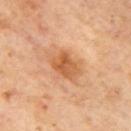<case>
  <biopsy_status>not biopsied; imaged during a skin examination</biopsy_status>
  <image>
    <source>total-body photography crop</source>
    <field_of_view_mm>15</field_of_view_mm>
  </image>
  <lesion_size>
    <long_diameter_mm_approx>4.0</long_diameter_mm_approx>
  </lesion_size>
  <site>right upper arm</site>
  <patient>
    <sex>female</sex>
    <age_approx>45</age_approx>
  </patient>
  <automated_metrics>
    <area_mm2_approx>9.5</area_mm2_approx>
    <eccentricity>0.65</eccentricity>
    <border_irregularity_0_10>2.0</border_irregularity_0_10>
    <color_variation_0_10>5.0</color_variation_0_10>
    <nevus_likeness_0_100>60</nevus_likeness_0_100>
    <lesion_detection_confidence_0_100>100</lesion_detection_confidence_0_100>
  </automated_metrics>
  <lighting>cross-polarized</lighting>
</case>